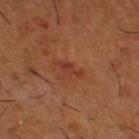<tbp_lesion>
<biopsy_status>not biopsied; imaged during a skin examination</biopsy_status>
</tbp_lesion>